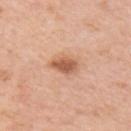follow-up: total-body-photography surveillance lesion; no biopsy
body site: the right upper arm
acquisition: ~15 mm tile from a whole-body skin photo
lesion size: ~3 mm (longest diameter)
TBP lesion metrics: an automated nevus-likeness rating near 95 out of 100 and lesion-presence confidence of about 100/100
subject: female, aged around 40